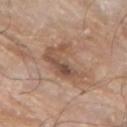A 15 mm close-up extracted from a 3D total-body photography capture. On the left forearm. The tile uses white-light illumination. The total-body-photography lesion software estimated a footprint of about 15 mm², an outline eccentricity of about 0.7 (0 = round, 1 = elongated), and a symmetry-axis asymmetry near 0.4. And it measured a lesion color around L≈52 a*≈18 b*≈29 in CIELAB and a lesion–skin lightness drop of about 9. The software also gave lesion-presence confidence of about 100/100. A male patient about 80 years old. Approximately 5 mm at its widest.